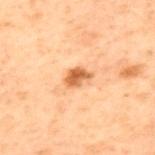No biopsy was performed on this lesion — it was imaged during a full skin examination and was not determined to be concerning.
A 15 mm crop from a total-body photograph taken for skin-cancer surveillance.
A male patient approximately 70 years of age.
The lesion is located on the back.